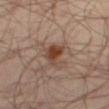Findings:
- biopsy status: no biopsy performed (imaged during a skin exam)
- illumination: cross-polarized
- subject: male, aged around 65
- site: the right thigh
- image: total-body-photography crop, ~15 mm field of view
- lesion diameter: ≈3 mm